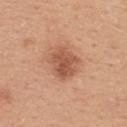{"biopsy_status": "not biopsied; imaged during a skin examination", "site": "upper back", "patient": {"sex": "female", "age_approx": 45}, "lesion_size": {"long_diameter_mm_approx": 4.0}, "image": {"source": "total-body photography crop", "field_of_view_mm": 15}, "automated_metrics": {"color_variation_0_10": 4.0}}